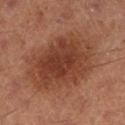Case summary:
- notes · imaged on a skin check; not biopsied
- body site · the left lower leg
- image · ~15 mm tile from a whole-body skin photo
- subject · male, in their 60s